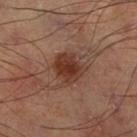No biopsy was performed on this lesion — it was imaged during a full skin examination and was not determined to be concerning. The tile uses cross-polarized illumination. The recorded lesion diameter is about 3.5 mm. Automated image analysis of the tile measured a footprint of about 11 mm² and a symmetry-axis asymmetry near 0.2. The analysis additionally found a lesion color around L≈38 a*≈22 b*≈28 in CIELAB and a lesion-to-skin contrast of about 9 (normalized; higher = more distinct). The lesion is on the leg. A lesion tile, about 15 mm wide, cut from a 3D total-body photograph.